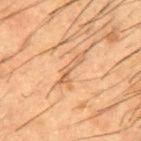follow-up: no biopsy performed (imaged during a skin exam) | TBP lesion metrics: an outline eccentricity of about 0.95 (0 = round, 1 = elongated) and two-axis asymmetry of about 0.5; border irregularity of about 7.5 on a 0–10 scale and radial color variation of about 0; a classifier nevus-likeness of about 0/100 and a lesion-detection confidence of about 60/100 | body site: the upper back | tile lighting: cross-polarized | image source: ~15 mm crop, total-body skin-cancer survey | subject: male, aged 48 to 52.